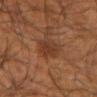Imaged during a routine full-body skin examination; the lesion was not biopsied and no histopathology is available.
A male subject, approximately 65 years of age.
The lesion is located on the arm.
Cropped from a total-body skin-imaging series; the visible field is about 15 mm.